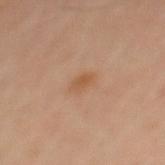The subject is a male in their 50s.
A lesion tile, about 15 mm wide, cut from a 3D total-body photograph.
Located on the mid back.